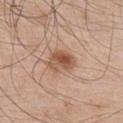Assessment: Captured during whole-body skin photography for melanoma surveillance; the lesion was not biopsied. Clinical summary: This image is a 15 mm lesion crop taken from a total-body photograph. Located on the chest. The patient is a male in their mid-40s.